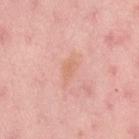Clinical impression: No biopsy was performed on this lesion — it was imaged during a full skin examination and was not determined to be concerning. Background: Imaged with white-light lighting. A female patient, aged approximately 50. From the lower back. The lesion's longest dimension is about 2.5 mm. Cropped from a whole-body photographic skin survey; the tile spans about 15 mm.